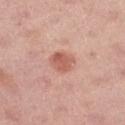The lesion was photographed on a routine skin check and not biopsied; there is no pathology result.
A female subject aged approximately 45.
The lesion is located on the left thigh.
This image is a 15 mm lesion crop taken from a total-body photograph.